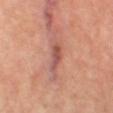workup — imaged on a skin check; not biopsied | subject — female, about 50 years old | anatomic site — the leg | automated lesion analysis — a footprint of about 5 mm², a shape eccentricity near 0.9, and a shape-asymmetry score of about 0.35 (0 = symmetric); an average lesion color of about L≈54 a*≈27 b*≈27 (CIELAB), roughly 10 lightness units darker than nearby skin, and a lesion-to-skin contrast of about 7 (normalized; higher = more distinct); an automated nevus-likeness rating near 0 out of 100 and lesion-presence confidence of about 85/100 | illumination — cross-polarized illumination | imaging modality — 15 mm crop, total-body photography.